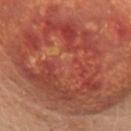notes = total-body-photography surveillance lesion; no biopsy | subject = female, aged approximately 50 | anatomic site = the chest | acquisition = ~15 mm crop, total-body skin-cancer survey.